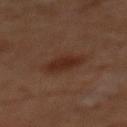Assessment:
Part of a total-body skin-imaging series; this lesion was reviewed on a skin check and was not flagged for biopsy.
Acquisition and patient details:
Measured at roughly 4 mm in maximum diameter. A male subject about 60 years old. The lesion is on the front of the torso. This image is a 15 mm lesion crop taken from a total-body photograph. Automated image analysis of the tile measured a lesion color around L≈25 a*≈18 b*≈23 in CIELAB, about 6 CIELAB-L* units darker than the surrounding skin, and a normalized border contrast of about 7.5.